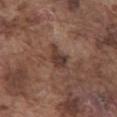Imaged during a routine full-body skin examination; the lesion was not biopsied and no histopathology is available. A roughly 15 mm field-of-view crop from a total-body skin photograph. An algorithmic analysis of the crop reported a footprint of about 5 mm². And it measured internal color variation of about 3 on a 0–10 scale and radial color variation of about 1. From the abdomen. This is a white-light tile. The patient is a male roughly 75 years of age.